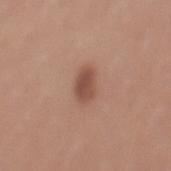follow-up: no biopsy performed (imaged during a skin exam)
anatomic site: the mid back
subject: male, aged 28 to 32
lighting: white-light illumination
imaging modality: ~15 mm tile from a whole-body skin photo